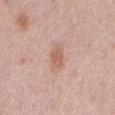Part of a total-body skin-imaging series; this lesion was reviewed on a skin check and was not flagged for biopsy. A roughly 15 mm field-of-view crop from a total-body skin photograph. The lesion's longest dimension is about 3 mm. Automated image analysis of the tile measured a shape eccentricity near 0.85 and a symmetry-axis asymmetry near 0.35. The analysis additionally found a mean CIELAB color near L≈60 a*≈22 b*≈28, about 9 CIELAB-L* units darker than the surrounding skin, and a lesion-to-skin contrast of about 6.5 (normalized; higher = more distinct). The analysis additionally found border irregularity of about 3 on a 0–10 scale. And it measured an automated nevus-likeness rating near 35 out of 100. A male patient, aged around 70. Imaged with white-light lighting. The lesion is located on the abdomen.